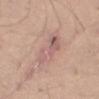notes: catalogued during a skin exam; not biopsied
image-analysis metrics: a mean CIELAB color near L≈61 a*≈20 b*≈23, roughly 8 lightness units darker than nearby skin, and a lesion-to-skin contrast of about 5.5 (normalized; higher = more distinct); a border-irregularity index near 3.5/10 and a within-lesion color-variation index near 7/10; a classifier nevus-likeness of about 0/100 and lesion-presence confidence of about 80/100
acquisition: ~15 mm tile from a whole-body skin photo
subject: male, aged approximately 25
body site: the leg
tile lighting: white-light